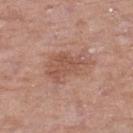<lesion>
  <biopsy_status>not biopsied; imaged during a skin examination</biopsy_status>
  <image>
    <source>total-body photography crop</source>
    <field_of_view_mm>15</field_of_view_mm>
  </image>
  <lighting>white-light</lighting>
  <automated_metrics>
    <border_irregularity_0_10>4.0</border_irregularity_0_10>
    <color_variation_0_10>2.5</color_variation_0_10>
    <peripheral_color_asymmetry>1.0</peripheral_color_asymmetry>
    <nevus_likeness_0_100>0</nevus_likeness_0_100>
    <lesion_detection_confidence_0_100>100</lesion_detection_confidence_0_100>
  </automated_metrics>
  <site>left lower leg</site>
  <lesion_size>
    <long_diameter_mm_approx>5.0</long_diameter_mm_approx>
  </lesion_size>
  <patient>
    <sex>female</sex>
    <age_approx>75</age_approx>
  </patient>
</lesion>